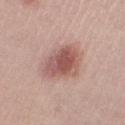notes: imaged on a skin check; not biopsied | image-analysis metrics: an area of roughly 14 mm², an eccentricity of roughly 0.85, and a symmetry-axis asymmetry near 0.15; a mean CIELAB color near L≈55 a*≈23 b*≈23, a lesion–skin lightness drop of about 13, and a normalized border contrast of about 8.5; a nevus-likeness score of about 90/100 and a detector confidence of about 100 out of 100 that the crop contains a lesion | illumination: white-light | diameter: ≈6 mm | imaging modality: 15 mm crop, total-body photography | patient: female, aged around 20 | anatomic site: the left thigh.